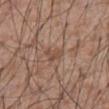{
  "biopsy_status": "not biopsied; imaged during a skin examination"
}